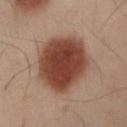Captured during whole-body skin photography for melanoma surveillance; the lesion was not biopsied.
The tile uses cross-polarized illumination.
Cropped from a whole-body photographic skin survey; the tile spans about 15 mm.
The lesion is on the left lower leg.
A male patient aged approximately 50.